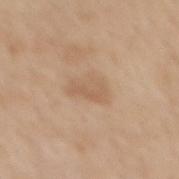notes: no biopsy performed (imaged during a skin exam) | body site: the mid back | diameter: ≈4 mm | acquisition: 15 mm crop, total-body photography | subject: female, in their mid-60s.